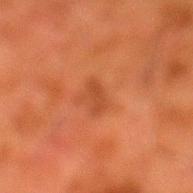Q: Illumination type?
A: cross-polarized illumination
Q: What is the lesion's diameter?
A: ~3 mm (longest diameter)
Q: What is the imaging modality?
A: ~15 mm tile from a whole-body skin photo
Q: Lesion location?
A: the left lower leg
Q: What are the patient's age and sex?
A: male, about 80 years old
Q: What did automated image analysis measure?
A: a lesion–skin lightness drop of about 7 and a normalized border contrast of about 5.5; a within-lesion color-variation index near 0/10 and peripheral color asymmetry of about 0; a lesion-detection confidence of about 100/100